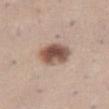  lesion_size:
    long_diameter_mm_approx: 4.5
  image:
    source: total-body photography crop
    field_of_view_mm: 15
  lighting: white-light
  site: abdomen
  patient:
    sex: female
    age_approx: 45
  automated_metrics:
    border_irregularity_0_10: 1.5
    color_variation_0_10: 7.0
    peripheral_color_asymmetry: 2.0
    nevus_likeness_0_100: 100
    lesion_detection_confidence_0_100: 100